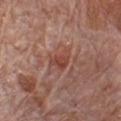– lesion size · about 3 mm
– anatomic site · the chest
– image source · ~15 mm tile from a whole-body skin photo
– lighting · white-light illumination
– automated lesion analysis · an average lesion color of about L≈45 a*≈25 b*≈27 (CIELAB) and about 8 CIELAB-L* units darker than the surrounding skin; a within-lesion color-variation index near 3/10 and peripheral color asymmetry of about 1; a detector confidence of about 100 out of 100 that the crop contains a lesion
– patient · male, roughly 80 years of age